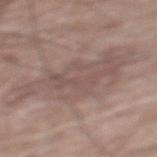A male patient, aged 58–62.
The lesion is located on the mid back.
Measured at roughly 12 mm in maximum diameter.
A lesion tile, about 15 mm wide, cut from a 3D total-body photograph.